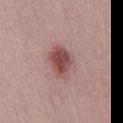Acquisition and patient details:
Cropped from a whole-body photographic skin survey; the tile spans about 15 mm. A male patient approximately 30 years of age. Longest diameter approximately 4.5 mm. The tile uses white-light illumination. The total-body-photography lesion software estimated an eccentricity of roughly 0.75 and a symmetry-axis asymmetry near 0.2. The software also gave border irregularity of about 2 on a 0–10 scale, internal color variation of about 5 on a 0–10 scale, and a peripheral color-asymmetry measure near 1.5.